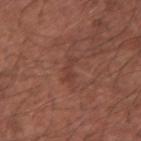workup: imaged on a skin check; not biopsied.